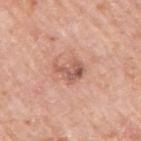This lesion was catalogued during total-body skin photography and was not selected for biopsy.
A region of skin cropped from a whole-body photographic capture, roughly 15 mm wide.
Imaged with white-light lighting.
Longest diameter approximately 3.5 mm.
An algorithmic analysis of the crop reported an area of roughly 6 mm² and a symmetry-axis asymmetry near 0.5. It also reported an average lesion color of about L≈58 a*≈23 b*≈28 (CIELAB). The analysis additionally found internal color variation of about 5.5 on a 0–10 scale.
The patient is a male aged 78–82.
The lesion is located on the right upper arm.